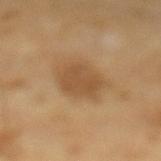{"biopsy_status": "not biopsied; imaged during a skin examination", "image": {"source": "total-body photography crop", "field_of_view_mm": 15}, "lighting": "cross-polarized", "lesion_size": {"long_diameter_mm_approx": 5.0}, "site": "left lower leg", "patient": {"sex": "male", "age_approx": 55}}